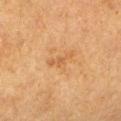Clinical impression:
Recorded during total-body skin imaging; not selected for excision or biopsy.
Context:
About 3.5 mm across. A region of skin cropped from a whole-body photographic capture, roughly 15 mm wide. The subject is a female aged 38–42. Located on the left lower leg.